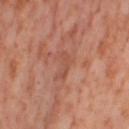* notes: catalogued during a skin exam; not biopsied
* subject: female, in their mid-50s
* imaging modality: ~15 mm tile from a whole-body skin photo
* site: the leg
* tile lighting: cross-polarized